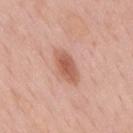Assessment: The lesion was photographed on a routine skin check and not biopsied; there is no pathology result. Clinical summary: A region of skin cropped from a whole-body photographic capture, roughly 15 mm wide. The patient is a male aged 53–57. This is a white-light tile. The lesion is located on the mid back. The lesion-visualizer software estimated an area of roughly 7.5 mm², a shape eccentricity near 0.85, and a shape-asymmetry score of about 0.2 (0 = symmetric). The software also gave a lesion color around L≈58 a*≈25 b*≈30 in CIELAB, a lesion–skin lightness drop of about 11, and a normalized border contrast of about 8. The software also gave border irregularity of about 2 on a 0–10 scale, a color-variation rating of about 2.5/10, and radial color variation of about 1. The analysis additionally found a nevus-likeness score of about 90/100 and a detector confidence of about 100 out of 100 that the crop contains a lesion.